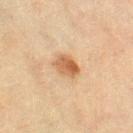Clinical impression:
Recorded during total-body skin imaging; not selected for excision or biopsy.
Image and clinical context:
The lesion's longest dimension is about 3 mm. On the right thigh. Cropped from a total-body skin-imaging series; the visible field is about 15 mm. An algorithmic analysis of the crop reported a lesion area of about 6 mm² and a shape-asymmetry score of about 0.25 (0 = symmetric). The software also gave a lesion color around L≈52 a*≈19 b*≈34 in CIELAB, roughly 11 lightness units darker than nearby skin, and a normalized lesion–skin contrast near 8.5. The software also gave a border-irregularity rating of about 2.5/10, a color-variation rating of about 4.5/10, and peripheral color asymmetry of about 1.5. The tile uses cross-polarized illumination. A female subject, aged around 80.